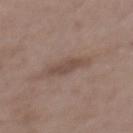Q: Was this lesion biopsied?
A: catalogued during a skin exam; not biopsied
Q: Where on the body is the lesion?
A: the mid back
Q: What kind of image is this?
A: 15 mm crop, total-body photography
Q: Who is the patient?
A: male, aged 48–52
Q: What is the lesion's diameter?
A: ~4 mm (longest diameter)